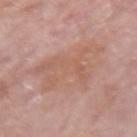The lesion was tiled from a total-body skin photograph and was not biopsied.
Captured under white-light illumination.
The recorded lesion diameter is about 8 mm.
On the right forearm.
The total-body-photography lesion software estimated a lesion area of about 26 mm² and two-axis asymmetry of about 0.4.
A female subject, aged around 65.
Cropped from a total-body skin-imaging series; the visible field is about 15 mm.